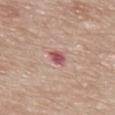Case summary:
– biopsy status · total-body-photography surveillance lesion; no biopsy
– lesion diameter · ≈3 mm
– illumination · white-light illumination
– patient · male, in their mid-80s or older
– body site · the upper back
– acquisition · total-body-photography crop, ~15 mm field of view